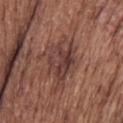follow-up = total-body-photography surveillance lesion; no biopsy.